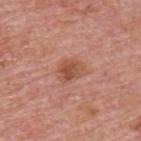<lesion>
  <biopsy_status>not biopsied; imaged during a skin examination</biopsy_status>
  <site>upper back</site>
  <image>
    <source>total-body photography crop</source>
    <field_of_view_mm>15</field_of_view_mm>
  </image>
  <patient>
    <sex>male</sex>
    <age_approx>75</age_approx>
  </patient>
</lesion>